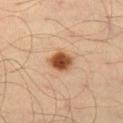- follow-up · imaged on a skin check; not biopsied
- image · 15 mm crop, total-body photography
- body site · the left thigh
- subject · male, aged 33 to 37